The subject is a male aged approximately 55; a close-up tile cropped from a whole-body skin photograph, about 15 mm across; the lesion is located on the arm.
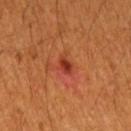The biopsy diagnosis was an invasive melanoma, superficial spreading type (Breslow thickness 0.32 mm, mitotic rate 0/mm²) — a skin cancer.